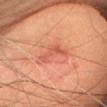Clinical impression: Imaged during a routine full-body skin examination; the lesion was not biopsied and no histopathology is available. Clinical summary: The lesion-visualizer software estimated a lesion area of about 4.5 mm², an outline eccentricity of about 0.95 (0 = round, 1 = elongated), and a shape-asymmetry score of about 0.5 (0 = symmetric). The analysis additionally found a border-irregularity rating of about 6.5/10 and a within-lesion color-variation index near 0.5/10. A female subject, approximately 60 years of age. A roughly 15 mm field-of-view crop from a total-body skin photograph. On the head or neck. This is a cross-polarized tile. Approximately 4 mm at its widest.